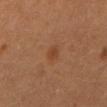Q: Was a biopsy performed?
A: total-body-photography surveillance lesion; no biopsy
Q: Lesion size?
A: ~2.5 mm (longest diameter)
Q: Patient demographics?
A: male, roughly 60 years of age
Q: Where on the body is the lesion?
A: the mid back
Q: How was this image acquired?
A: ~15 mm tile from a whole-body skin photo